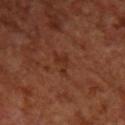| field | value |
|---|---|
| follow-up | catalogued during a skin exam; not biopsied |
| subject | female, about 65 years old |
| imaging modality | total-body-photography crop, ~15 mm field of view |
| body site | the right forearm |
| tile lighting | cross-polarized illumination |
| size | ≈3 mm |